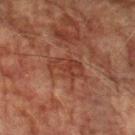Acquisition and patient details:
Automated tile analysis of the lesion measured a footprint of about 7.5 mm², an outline eccentricity of about 0.8 (0 = round, 1 = elongated), and a symmetry-axis asymmetry near 0.35. The analysis additionally found a lesion color around L≈32 a*≈22 b*≈26 in CIELAB, roughly 6 lightness units darker than nearby skin, and a normalized border contrast of about 6. Cropped from a whole-body photographic skin survey; the tile spans about 15 mm. The lesion is on the left forearm. The subject is a male about 75 years old. The tile uses cross-polarized illumination.